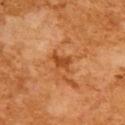Recorded during total-body skin imaging; not selected for excision or biopsy. The recorded lesion diameter is about 3 mm. Automated tile analysis of the lesion measured an average lesion color of about L≈50 a*≈30 b*≈45 (CIELAB). The analysis additionally found a border-irregularity index near 4/10, a within-lesion color-variation index near 1/10, and radial color variation of about 0.5. A female patient aged around 55. The lesion is located on the upper back. A roughly 15 mm field-of-view crop from a total-body skin photograph.